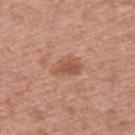A male patient, aged around 60.
A lesion tile, about 15 mm wide, cut from a 3D total-body photograph.
An algorithmic analysis of the crop reported a border-irregularity rating of about 2.5/10 and a color-variation rating of about 2.5/10. And it measured a classifier nevus-likeness of about 65/100.
Captured under white-light illumination.
Located on the upper back.
The recorded lesion diameter is about 3.5 mm.